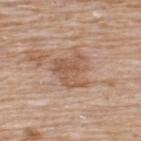{
  "biopsy_status": "not biopsied; imaged during a skin examination",
  "image": {
    "source": "total-body photography crop",
    "field_of_view_mm": 15
  },
  "lighting": "white-light",
  "site": "upper back",
  "lesion_size": {
    "long_diameter_mm_approx": 4.0
  },
  "patient": {
    "sex": "male",
    "age_approx": 80
  }
}